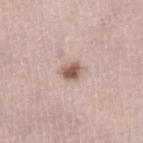Notes:
* workup: catalogued during a skin exam; not biopsied
* lighting: white-light illumination
* patient: female, aged 48 to 52
* imaging modality: ~15 mm tile from a whole-body skin photo
* size: about 2.5 mm
* anatomic site: the left lower leg